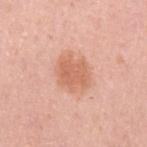workup = total-body-photography surveillance lesion; no biopsy
subject = female, about 30 years old
tile lighting = white-light
anatomic site = the arm
lesion size = about 4 mm
automated lesion analysis = an area of roughly 11 mm² and two-axis asymmetry of about 0.2; a lesion color around L≈64 a*≈26 b*≈33 in CIELAB, about 9 CIELAB-L* units darker than the surrounding skin, and a normalized lesion–skin contrast near 6.5; border irregularity of about 2.5 on a 0–10 scale, internal color variation of about 2 on a 0–10 scale, and a peripheral color-asymmetry measure near 0.5
image source = ~15 mm tile from a whole-body skin photo